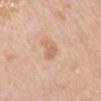Impression:
This lesion was catalogued during total-body skin photography and was not selected for biopsy.
Clinical summary:
This image is a 15 mm lesion crop taken from a total-body photograph. The lesion's longest dimension is about 3.5 mm. The lesion-visualizer software estimated an average lesion color of about L≈65 a*≈19 b*≈32 (CIELAB), roughly 9 lightness units darker than nearby skin, and a normalized lesion–skin contrast near 5.5. The analysis additionally found a within-lesion color-variation index near 0.5/10. A male patient aged approximately 80. The lesion is located on the back.